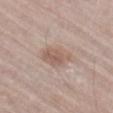follow-up: no biopsy performed (imaged during a skin exam) | automated metrics: a mean CIELAB color near L≈58 a*≈17 b*≈25, a lesion–skin lightness drop of about 9, and a lesion-to-skin contrast of about 6 (normalized; higher = more distinct); a nevus-likeness score of about 50/100 and a detector confidence of about 100 out of 100 that the crop contains a lesion | patient: male, about 80 years old | image: ~15 mm tile from a whole-body skin photo | anatomic site: the left thigh | diameter: about 4 mm.